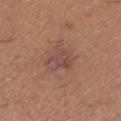notes — imaged on a skin check; not biopsied
TBP lesion metrics — a mean CIELAB color near L≈47 a*≈23 b*≈24, about 7 CIELAB-L* units darker than the surrounding skin, and a normalized border contrast of about 6; a border-irregularity index near 3/10; an automated nevus-likeness rating near 0 out of 100 and a lesion-detection confidence of about 100/100
lesion diameter — ≈3.5 mm
acquisition — ~15 mm tile from a whole-body skin photo
subject — male, roughly 40 years of age
location — the left lower leg
lighting — white-light